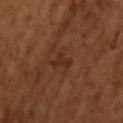Impression: No biopsy was performed on this lesion — it was imaged during a full skin examination and was not determined to be concerning. Acquisition and patient details: Imaged with cross-polarized lighting. The subject is a male approximately 65 years of age. Approximately 3 mm at its widest. A 15 mm close-up tile from a total-body photography series done for melanoma screening. The total-body-photography lesion software estimated an average lesion color of about L≈28 a*≈20 b*≈28 (CIELAB), about 6 CIELAB-L* units darker than the surrounding skin, and a normalized lesion–skin contrast near 6. The software also gave a nevus-likeness score of about 0/100 and a detector confidence of about 100 out of 100 that the crop contains a lesion. Located on the upper back.